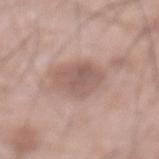Imaged during a routine full-body skin examination; the lesion was not biopsied and no histopathology is available. A 15 mm close-up extracted from a 3D total-body photography capture. About 4 mm across. The lesion is on the abdomen. The subject is a male aged 68–72. Captured under white-light illumination. Automated image analysis of the tile measured a footprint of about 11 mm² and a shape-asymmetry score of about 0.2 (0 = symmetric).